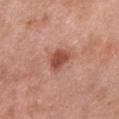Imaged during a routine full-body skin examination; the lesion was not biopsied and no histopathology is available. A male patient, in their mid- to late 50s. This image is a 15 mm lesion crop taken from a total-body photograph. From the left upper arm.